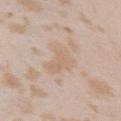Impression: Part of a total-body skin-imaging series; this lesion was reviewed on a skin check and was not flagged for biopsy. Image and clinical context: Located on the right upper arm. A female patient about 25 years old. A roughly 15 mm field-of-view crop from a total-body skin photograph.